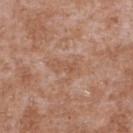Imaged during a routine full-body skin examination; the lesion was not biopsied and no histopathology is available. Longest diameter approximately 3 mm. The total-body-photography lesion software estimated roughly 6 lightness units darker than nearby skin and a normalized border contrast of about 5. The software also gave a border-irregularity index near 5.5/10, a within-lesion color-variation index near 0/10, and radial color variation of about 0. And it measured a nevus-likeness score of about 0/100 and a lesion-detection confidence of about 100/100. This image is a 15 mm lesion crop taken from a total-body photograph. On the upper back. This is a white-light tile. A male patient, aged approximately 45.